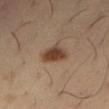Impression: Part of a total-body skin-imaging series; this lesion was reviewed on a skin check and was not flagged for biopsy. Acquisition and patient details: Approximately 3.5 mm at its widest. The subject is a male approximately 50 years of age. A 15 mm crop from a total-body photograph taken for skin-cancer surveillance. Imaged with cross-polarized lighting. Located on the right thigh.